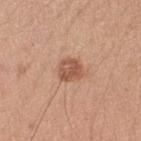<case>
<biopsy_status>not biopsied; imaged during a skin examination</biopsy_status>
<automated_metrics>
  <cielab_L>55</cielab_L>
  <cielab_a>23</cielab_a>
  <cielab_b>31</cielab_b>
  <vs_skin_darker_L>11.0</vs_skin_darker_L>
  <vs_skin_contrast_norm>7.5</vs_skin_contrast_norm>
  <border_irregularity_0_10>1.5</border_irregularity_0_10>
  <color_variation_0_10>4.0</color_variation_0_10>
  <peripheral_color_asymmetry>1.5</peripheral_color_asymmetry>
  <lesion_detection_confidence_0_100>100</lesion_detection_confidence_0_100>
</automated_metrics>
<site>arm</site>
<lesion_size>
  <long_diameter_mm_approx>3.0</long_diameter_mm_approx>
</lesion_size>
<image>
  <source>total-body photography crop</source>
  <field_of_view_mm>15</field_of_view_mm>
</image>
<patient>
  <sex>female</sex>
  <age_approx>45</age_approx>
</patient>
<lighting>white-light</lighting>
</case>